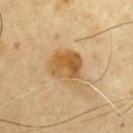notes: catalogued during a skin exam; not biopsied
location: the chest
lesion size: about 4.5 mm
subject: male, in their mid-60s
image source: ~15 mm tile from a whole-body skin photo